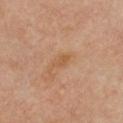Impression:
Captured during whole-body skin photography for melanoma surveillance; the lesion was not biopsied.
Image and clinical context:
The tile uses cross-polarized illumination. The recorded lesion diameter is about 2.5 mm. A 15 mm close-up tile from a total-body photography series done for melanoma screening. Automated image analysis of the tile measured a footprint of about 3 mm², an outline eccentricity of about 0.85 (0 = round, 1 = elongated), and two-axis asymmetry of about 0.2. The analysis additionally found border irregularity of about 2 on a 0–10 scale, a within-lesion color-variation index near 1.5/10, and radial color variation of about 0.5. A female patient aged 33 to 37. The lesion is on the chest.